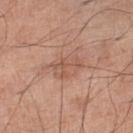Assessment:
The lesion was tiled from a total-body skin photograph and was not biopsied.
Acquisition and patient details:
Automated image analysis of the tile measured a lesion color around L≈55 a*≈22 b*≈29 in CIELAB, a lesion–skin lightness drop of about 7, and a normalized border contrast of about 5.5. The analysis additionally found a lesion-detection confidence of about 100/100. Located on the left lower leg. About 3.5 mm across. The tile uses white-light illumination. A male subject, aged 68–72. A close-up tile cropped from a whole-body skin photograph, about 15 mm across.